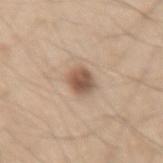No biopsy was performed on this lesion — it was imaged during a full skin examination and was not determined to be concerning. A male subject, in their 60s. The lesion is located on the right thigh. Cropped from a total-body skin-imaging series; the visible field is about 15 mm.